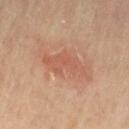Q: Was this lesion biopsied?
A: catalogued during a skin exam; not biopsied
Q: How was the tile lit?
A: cross-polarized
Q: What are the patient's age and sex?
A: female, aged around 75
Q: How was this image acquired?
A: total-body-photography crop, ~15 mm field of view
Q: What did automated image analysis measure?
A: an area of roughly 10 mm², a shape eccentricity near 0.85, and a shape-asymmetry score of about 0.4 (0 = symmetric); a border-irregularity index near 5/10 and peripheral color asymmetry of about 1; an automated nevus-likeness rating near 5 out of 100
Q: What is the anatomic site?
A: the right thigh